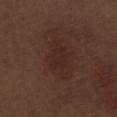Q: Was this lesion biopsied?
A: catalogued during a skin exam; not biopsied
Q: What is the imaging modality?
A: 15 mm crop, total-body photography
Q: What is the lesion's diameter?
A: ~3.5 mm (longest diameter)
Q: Automated lesion metrics?
A: a lesion area of about 5.5 mm² and a shape eccentricity near 0.75; a lesion-detection confidence of about 100/100
Q: What are the patient's age and sex?
A: male, about 70 years old
Q: Lesion location?
A: the left thigh
Q: Illumination type?
A: white-light